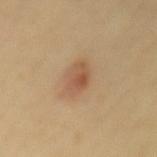| key | value |
|---|---|
| TBP lesion metrics | an area of roughly 6.5 mm² and an eccentricity of roughly 0.85; a lesion color around L≈55 a*≈20 b*≈34 in CIELAB, a lesion–skin lightness drop of about 10, and a normalized lesion–skin contrast near 6.5; border irregularity of about 2 on a 0–10 scale, internal color variation of about 4 on a 0–10 scale, and radial color variation of about 1; a classifier nevus-likeness of about 95/100 and lesion-presence confidence of about 100/100 |
| lesion size | about 4 mm |
| image source | 15 mm crop, total-body photography |
| tile lighting | cross-polarized |
| patient | female, in their 60s |
| site | the mid back |